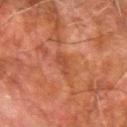Impression:
Recorded during total-body skin imaging; not selected for excision or biopsy.
Background:
From the arm. The patient is a male aged around 80. A 15 mm close-up extracted from a 3D total-body photography capture. This is a cross-polarized tile.